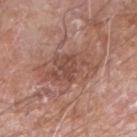Context: Longest diameter approximately 6.5 mm. Located on the right forearm. A male subject aged approximately 60. This is a white-light tile. Cropped from a whole-body photographic skin survey; the tile spans about 15 mm.